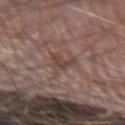No biopsy was performed on this lesion — it was imaged during a full skin examination and was not determined to be concerning. The lesion-visualizer software estimated border irregularity of about 6 on a 0–10 scale, a within-lesion color-variation index near 2/10, and radial color variation of about 0.5. The analysis additionally found a detector confidence of about 95 out of 100 that the crop contains a lesion. The subject is a male aged 73 to 77. From the left forearm. Longest diameter approximately 3 mm. The tile uses white-light illumination. A close-up tile cropped from a whole-body skin photograph, about 15 mm across.